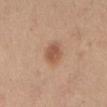Image and clinical context: This image is a 15 mm lesion crop taken from a total-body photograph. A female subject aged around 40. Located on the abdomen.